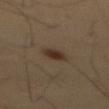Imaged during a routine full-body skin examination; the lesion was not biopsied and no histopathology is available. A male subject, aged around 55. A 15 mm close-up tile from a total-body photography series done for melanoma screening. From the abdomen.